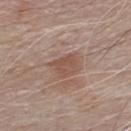tile lighting = white-light illumination
lesion diameter = ~3.5 mm (longest diameter)
site = the chest
acquisition = 15 mm crop, total-body photography
subject = male, in their 70s
automated metrics = an area of roughly 6 mm², an eccentricity of roughly 0.65, and a symmetry-axis asymmetry near 0.4; a mean CIELAB color near L≈51 a*≈19 b*≈26, roughly 8 lightness units darker than nearby skin, and a normalized lesion–skin contrast near 6.5; an automated nevus-likeness rating near 5 out of 100 and a lesion-detection confidence of about 100/100